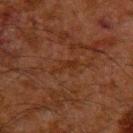Q: Was a biopsy performed?
A: imaged on a skin check; not biopsied
Q: What kind of image is this?
A: ~15 mm tile from a whole-body skin photo
Q: What is the anatomic site?
A: the upper back
Q: What lighting was used for the tile?
A: cross-polarized illumination
Q: What are the patient's age and sex?
A: male, in their 60s
Q: What is the lesion's diameter?
A: ≈3.5 mm